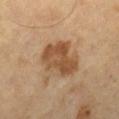| field | value |
|---|---|
| acquisition | ~15 mm crop, total-body skin-cancer survey |
| lesion size | ≈5.5 mm |
| lighting | cross-polarized illumination |
| patient | male, aged approximately 65 |